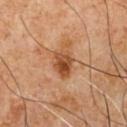Part of a total-body skin-imaging series; this lesion was reviewed on a skin check and was not flagged for biopsy. From the chest. This image is a 15 mm lesion crop taken from a total-body photograph. The lesion's longest dimension is about 3 mm. A male subject approximately 60 years of age.